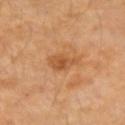A female subject aged 58–62.
From the left forearm.
A 15 mm crop from a total-body photograph taken for skin-cancer surveillance.
About 3 mm across.
The tile uses cross-polarized illumination.